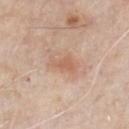follow-up = total-body-photography surveillance lesion; no biopsy